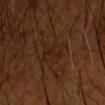biopsy status = no biopsy performed (imaged during a skin exam)
size = about 3.5 mm
body site = the left forearm
patient = male, approximately 65 years of age
image = ~15 mm tile from a whole-body skin photo
tile lighting = cross-polarized